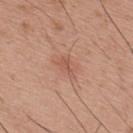workup = total-body-photography surveillance lesion; no biopsy
body site = the upper back
tile lighting = white-light
diameter = ~3 mm (longest diameter)
subject = male, aged approximately 30
imaging modality = ~15 mm crop, total-body skin-cancer survey